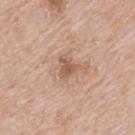notes = imaged on a skin check; not biopsied.